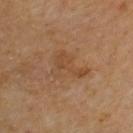Part of a total-body skin-imaging series; this lesion was reviewed on a skin check and was not flagged for biopsy. The lesion's longest dimension is about 4.5 mm. Imaged with cross-polarized lighting. A 15 mm close-up extracted from a 3D total-body photography capture. Automated tile analysis of the lesion measured a footprint of about 9 mm², a shape eccentricity near 0.75, and a symmetry-axis asymmetry near 0.6. And it measured border irregularity of about 6.5 on a 0–10 scale, a color-variation rating of about 2.5/10, and peripheral color asymmetry of about 1. The analysis additionally found a nevus-likeness score of about 0/100 and lesion-presence confidence of about 100/100. From the left upper arm. The patient is a male aged around 70.